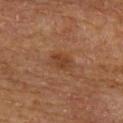The lesion was photographed on a routine skin check and not biopsied; there is no pathology result.
Automated image analysis of the tile measured a shape eccentricity near 0.6 and a shape-asymmetry score of about 0.2 (0 = symmetric). It also reported an average lesion color of about L≈36 a*≈20 b*≈30 (CIELAB), about 7 CIELAB-L* units darker than the surrounding skin, and a normalized border contrast of about 6.5. It also reported a border-irregularity index near 2/10, a within-lesion color-variation index near 1.5/10, and a peripheral color-asymmetry measure near 0.5.
Cropped from a total-body skin-imaging series; the visible field is about 15 mm.
The tile uses cross-polarized illumination.
A male subject aged 63 to 67.
On the upper back.
The recorded lesion diameter is about 2.5 mm.